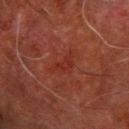biopsy_status: not biopsied; imaged during a skin examination
image:
  source: total-body photography crop
  field_of_view_mm: 15
site: arm
lesion_size:
  long_diameter_mm_approx: 4.5
patient:
  sex: male
  age_approx: 80